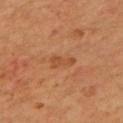Notes:
- notes — catalogued during a skin exam; not biopsied
- lesion diameter — about 3 mm
- anatomic site — the back
- patient — male, in their mid- to late 50s
- acquisition — total-body-photography crop, ~15 mm field of view
- lighting — cross-polarized illumination